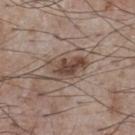Assessment: Recorded during total-body skin imaging; not selected for excision or biopsy. Image and clinical context: The lesion is located on the chest. A male subject, aged 73–77. Automated image analysis of the tile measured a footprint of about 9 mm², an eccentricity of roughly 0.85, and a symmetry-axis asymmetry near 0.35. It also reported a mean CIELAB color near L≈45 a*≈16 b*≈24, about 12 CIELAB-L* units darker than the surrounding skin, and a lesion-to-skin contrast of about 9 (normalized; higher = more distinct). The software also gave border irregularity of about 4 on a 0–10 scale, a color-variation rating of about 4.5/10, and peripheral color asymmetry of about 1.5. Captured under white-light illumination. Approximately 4.5 mm at its widest. A close-up tile cropped from a whole-body skin photograph, about 15 mm across.